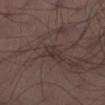workup — total-body-photography surveillance lesion; no biopsy